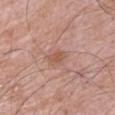This lesion was catalogued during total-body skin photography and was not selected for biopsy.
From the right upper arm.
The tile uses white-light illumination.
The subject is a male approximately 65 years of age.
The lesion's longest dimension is about 2.5 mm.
This image is a 15 mm lesion crop taken from a total-body photograph.